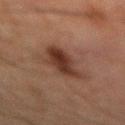No biopsy was performed on this lesion — it was imaged during a full skin examination and was not determined to be concerning. A lesion tile, about 15 mm wide, cut from a 3D total-body photograph. Automated tile analysis of the lesion measured a lesion color around L≈27 a*≈17 b*≈22 in CIELAB, a lesion–skin lightness drop of about 10, and a normalized border contrast of about 10. The analysis additionally found a border-irregularity rating of about 3/10 and a color-variation rating of about 3.5/10. The software also gave a classifier nevus-likeness of about 90/100 and a lesion-detection confidence of about 100/100. A male patient in their mid-60s. The lesion is located on the back.